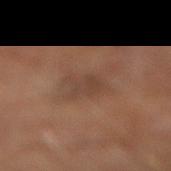follow-up = total-body-photography surveillance lesion; no biopsy | diameter = ≈3 mm | imaging modality = ~15 mm crop, total-body skin-cancer survey | patient = male, approximately 60 years of age | body site = the leg | lighting = cross-polarized.